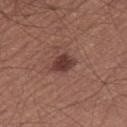Part of a total-body skin-imaging series; this lesion was reviewed on a skin check and was not flagged for biopsy.